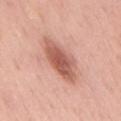biopsy status: total-body-photography surveillance lesion; no biopsy | image: total-body-photography crop, ~15 mm field of view | subject: male, in their mid-50s | illumination: white-light | TBP lesion metrics: a footprint of about 14 mm², an outline eccentricity of about 0.9 (0 = round, 1 = elongated), and a symmetry-axis asymmetry near 0.15; a lesion–skin lightness drop of about 14 and a normalized lesion–skin contrast near 9; a border-irregularity index near 2.5/10, internal color variation of about 4.5 on a 0–10 scale, and peripheral color asymmetry of about 1.5 | body site: the mid back | lesion size: ~7 mm (longest diameter).